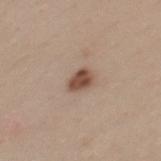Assessment:
This lesion was catalogued during total-body skin photography and was not selected for biopsy.
Clinical summary:
From the upper back. Cropped from a total-body skin-imaging series; the visible field is about 15 mm. This is a white-light tile. A female subject about 25 years old.